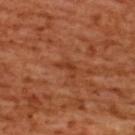<lesion>
  <biopsy_status>not biopsied; imaged during a skin examination</biopsy_status>
  <automated_metrics>
    <vs_skin_darker_L>7.0</vs_skin_darker_L>
    <vs_skin_contrast_norm>6.5</vs_skin_contrast_norm>
    <nevus_likeness_0_100>0</nevus_likeness_0_100>
    <lesion_detection_confidence_0_100>95</lesion_detection_confidence_0_100>
  </automated_metrics>
  <site>upper back</site>
  <image>
    <source>total-body photography crop</source>
    <field_of_view_mm>15</field_of_view_mm>
  </image>
  <lesion_size>
    <long_diameter_mm_approx>2.5</long_diameter_mm_approx>
  </lesion_size>
  <lighting>cross-polarized</lighting>
  <patient>
    <sex>female</sex>
    <age_approx>55</age_approx>
  </patient>
</lesion>